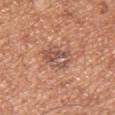Imaged during a routine full-body skin examination; the lesion was not biopsied and no histopathology is available.
The patient is a male roughly 30 years of age.
From the left upper arm.
Captured under white-light illumination.
A region of skin cropped from a whole-body photographic capture, roughly 15 mm wide.
About 3.5 mm across.
Automated image analysis of the tile measured an area of roughly 9.5 mm² and an eccentricity of roughly 0.6. It also reported an average lesion color of about L≈54 a*≈22 b*≈29 (CIELAB), about 9 CIELAB-L* units darker than the surrounding skin, and a lesion-to-skin contrast of about 6.5 (normalized; higher = more distinct). The analysis additionally found a within-lesion color-variation index near 7/10 and peripheral color asymmetry of about 2.5.